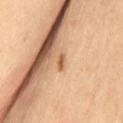Part of a total-body skin-imaging series; this lesion was reviewed on a skin check and was not flagged for biopsy. A female subject, aged 63 to 67. A 15 mm close-up tile from a total-body photography series done for melanoma screening. From the left thigh.